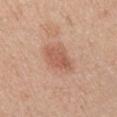  biopsy_status: not biopsied; imaged during a skin examination
  automated_metrics:
    area_mm2_approx: 8.5
    eccentricity: 0.75
    border_irregularity_0_10: 2.0
    color_variation_0_10: 3.0
    peripheral_color_asymmetry: 1.0
    nevus_likeness_0_100: 90
  lighting: white-light
  patient:
    sex: male
    age_approx: 55
  image:
    source: total-body photography crop
    field_of_view_mm: 15
  lesion_size:
    long_diameter_mm_approx: 4.0
  site: arm